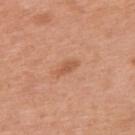The lesion was photographed on a routine skin check and not biopsied; there is no pathology result. Captured under white-light illumination. Located on the upper back. A lesion tile, about 15 mm wide, cut from a 3D total-body photograph. A male patient, roughly 70 years of age. Longest diameter approximately 2.5 mm.